On the upper back · cropped from a whole-body photographic skin survey; the tile spans about 15 mm · a female patient, about 50 years old: 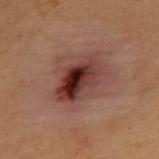<record>
<lighting>cross-polarized</lighting>
<automated_metrics>
  <cielab_L>29</cielab_L>
  <cielab_a>21</cielab_a>
  <cielab_b>21</cielab_b>
  <vs_skin_darker_L>12.0</vs_skin_darker_L>
  <vs_skin_contrast_norm>11.5</vs_skin_contrast_norm>
  <nevus_likeness_0_100>35</nevus_likeness_0_100>
  <lesion_detection_confidence_0_100>100</lesion_detection_confidence_0_100>
</automated_metrics>
<lesion_size>
  <long_diameter_mm_approx>5.5</long_diameter_mm_approx>
</lesion_size>
<diagnosis>
  <histopathology>atypical intraepithelial melanocytic proliferation</histopathology>
  <malignancy>indeterminate</malignancy>
  <taxonomic_path>Indeterminate, Indeterminate melanocytic proliferations, Atypical intraepithelial melanocytic proliferation</taxonomic_path>
</diagnosis>
</record>